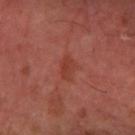workup: catalogued during a skin exam; not biopsied
illumination: cross-polarized
site: the left forearm
patient: aged approximately 65
image: 15 mm crop, total-body photography